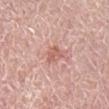This lesion was catalogued during total-body skin photography and was not selected for biopsy. A female patient in their mid-50s. Cropped from a whole-body photographic skin survey; the tile spans about 15 mm. From the right lower leg.